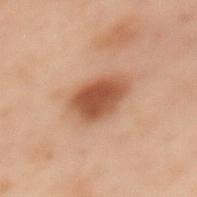| key | value |
|---|---|
| biopsy status | imaged on a skin check; not biopsied |
| patient | female, aged 53 to 57 |
| image | total-body-photography crop, ~15 mm field of view |
| automated lesion analysis | an area of roughly 12 mm²; an average lesion color of about L≈43 a*≈20 b*≈29 (CIELAB), roughly 12 lightness units darker than nearby skin, and a lesion-to-skin contrast of about 9.5 (normalized; higher = more distinct) |
| anatomic site | the mid back |
| tile lighting | cross-polarized illumination |
| diameter | ~4.5 mm (longest diameter) |